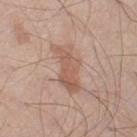Background: The patient is a male aged 68 to 72. About 5.5 mm across. The lesion is located on the right thigh. The lesion-visualizer software estimated a footprint of about 11 mm² and a shape eccentricity near 0.9. The analysis additionally found roughly 9 lightness units darker than nearby skin. It also reported a border-irregularity rating of about 4.5/10, a within-lesion color-variation index near 3/10, and peripheral color asymmetry of about 1. It also reported an automated nevus-likeness rating near 5 out of 100 and lesion-presence confidence of about 100/100. A region of skin cropped from a whole-body photographic capture, roughly 15 mm wide.